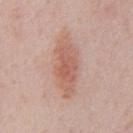{"biopsy_status": "not biopsied; imaged during a skin examination"}